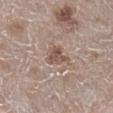<lesion>
  <biopsy_status>not biopsied; imaged during a skin examination</biopsy_status>
  <patient>
    <sex>female</sex>
    <age_approx>50</age_approx>
  </patient>
  <image>
    <source>total-body photography crop</source>
    <field_of_view_mm>15</field_of_view_mm>
  </image>
  <site>right lower leg</site>
</lesion>